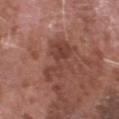{
  "biopsy_status": "not biopsied; imaged during a skin examination",
  "lesion_size": {
    "long_diameter_mm_approx": 6.0
  },
  "automated_metrics": {
    "shape_asymmetry": 0.5,
    "vs_skin_darker_L": 8.0,
    "nevus_likeness_0_100": 0,
    "lesion_detection_confidence_0_100": 100
  },
  "image": {
    "source": "total-body photography crop",
    "field_of_view_mm": 15
  },
  "site": "head or neck",
  "patient": {
    "sex": "male",
    "age_approx": 75
  }
}